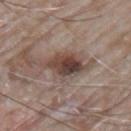The lesion was tiled from a total-body skin photograph and was not biopsied. The lesion's longest dimension is about 5 mm. Captured under white-light illumination. This image is a 15 mm lesion crop taken from a total-body photograph. On the left upper arm. A male subject aged around 65.